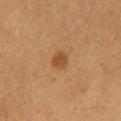Q: Is there a histopathology result?
A: catalogued during a skin exam; not biopsied
Q: How was this image acquired?
A: total-body-photography crop, ~15 mm field of view
Q: What is the anatomic site?
A: the mid back
Q: What are the patient's age and sex?
A: female, roughly 40 years of age